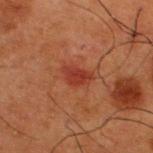The lesion was photographed on a routine skin check and not biopsied; there is no pathology result. The tile uses cross-polarized illumination. About 3.5 mm across. The subject is a male aged approximately 50. Cropped from a whole-body photographic skin survey; the tile spans about 15 mm. From the upper back.